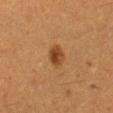biopsy_status: not biopsied; imaged during a skin examination
image:
  source: total-body photography crop
  field_of_view_mm: 15
patient:
  sex: female
  age_approx: 30
site: right leg